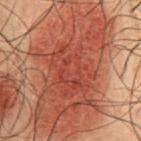  biopsy_status: not biopsied; imaged during a skin examination
  patient:
    sex: male
    age_approx: 50
  automated_metrics:
    vs_skin_darker_L: 9.0
    vs_skin_contrast_norm: 8.0
    border_irregularity_0_10: 3.5
    peripheral_color_asymmetry: 1.5
    lesion_detection_confidence_0_100: 65
  lesion_size:
    long_diameter_mm_approx: 15.5
  lighting: cross-polarized
  site: abdomen
  image:
    source: total-body photography crop
    field_of_view_mm: 15